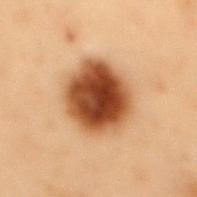| key | value |
|---|---|
| imaging modality | ~15 mm crop, total-body skin-cancer survey |
| tile lighting | cross-polarized illumination |
| subject | male, aged 53 to 57 |
| anatomic site | the mid back |
| TBP lesion metrics | a lesion area of about 26 mm² and a shape-asymmetry score of about 0.1 (0 = symmetric); an average lesion color of about L≈40 a*≈21 b*≈32 (CIELAB) and roughly 18 lightness units darker than nearby skin; a within-lesion color-variation index near 7.5/10; a classifier nevus-likeness of about 100/100 |
| lesion diameter | ≈6 mm |